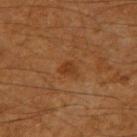Captured during whole-body skin photography for melanoma surveillance; the lesion was not biopsied.
This image is a 15 mm lesion crop taken from a total-body photograph.
Automated tile analysis of the lesion measured a shape eccentricity near 0.65 and two-axis asymmetry of about 0.25. And it measured a within-lesion color-variation index near 1/10 and peripheral color asymmetry of about 0. The software also gave a nevus-likeness score of about 45/100 and a detector confidence of about 100 out of 100 that the crop contains a lesion.
The subject is a male roughly 60 years of age.
The lesion's longest dimension is about 2 mm.
The tile uses cross-polarized illumination.
On the right upper arm.